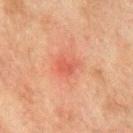The lesion was tiled from a total-body skin photograph and was not biopsied.
A 15 mm close-up tile from a total-body photography series done for melanoma screening.
Automated image analysis of the tile measured a footprint of about 4 mm², an eccentricity of roughly 0.65, and a symmetry-axis asymmetry near 0.3. And it measured a border-irregularity index near 3/10.
Located on the chest.
A male subject, aged 73 to 77.
Longest diameter approximately 2.5 mm.